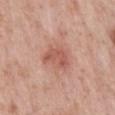The lesion was tiled from a total-body skin photograph and was not biopsied. The lesion is on the back. Approximately 4 mm at its widest. The tile uses white-light illumination. The subject is a male roughly 65 years of age. Automated tile analysis of the lesion measured a lesion area of about 7 mm², an outline eccentricity of about 0.75 (0 = round, 1 = elongated), and two-axis asymmetry of about 0.35. It also reported a normalized border contrast of about 6.5. It also reported lesion-presence confidence of about 100/100. A 15 mm crop from a total-body photograph taken for skin-cancer surveillance.